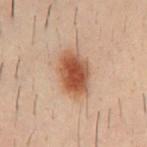Case summary:
* biopsy status — total-body-photography surveillance lesion; no biopsy
* tile lighting — cross-polarized
* patient — male, aged approximately 30
* diameter — about 5 mm
* image source — 15 mm crop, total-body photography
* automated metrics — a footprint of about 14 mm², a shape eccentricity near 0.75, and two-axis asymmetry of about 0.1; internal color variation of about 5 on a 0–10 scale and a peripheral color-asymmetry measure near 1.5; a nevus-likeness score of about 100/100
* site — the chest